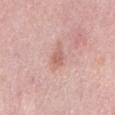biopsy_status: not biopsied; imaged during a skin examination
patient:
  sex: male
  age_approx: 55
site: back
automated_metrics:
  cielab_L: 62
  cielab_a: 24
  cielab_b: 27
  vs_skin_darker_L: 9.0
  border_irregularity_0_10: 3.0
  color_variation_0_10: 1.5
  peripheral_color_asymmetry: 0.5
lesion_size:
  long_diameter_mm_approx: 3.0
lighting: white-light
image:
  source: total-body photography crop
  field_of_view_mm: 15